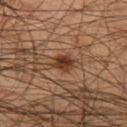Clinical impression:
The lesion was photographed on a routine skin check and not biopsied; there is no pathology result.
Context:
The recorded lesion diameter is about 3 mm. Located on the left thigh. Automated image analysis of the tile measured a footprint of about 4.5 mm² and an eccentricity of roughly 0.65. The analysis additionally found an average lesion color of about L≈35 a*≈20 b*≈29 (CIELAB) and a lesion-to-skin contrast of about 10 (normalized; higher = more distinct). A 15 mm close-up extracted from a 3D total-body photography capture. The subject is a male aged around 45.